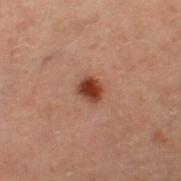Impression:
This lesion was catalogued during total-body skin photography and was not selected for biopsy.
Context:
Imaged with cross-polarized lighting. Located on the right thigh. The subject is a male about 60 years old. A 15 mm close-up extracted from a 3D total-body photography capture. The total-body-photography lesion software estimated a footprint of about 4.5 mm² and a symmetry-axis asymmetry near 0.15.